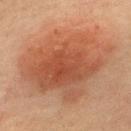<record>
  <biopsy_status>not biopsied; imaged during a skin examination</biopsy_status>
  <image>
    <source>total-body photography crop</source>
    <field_of_view_mm>15</field_of_view_mm>
  </image>
  <site>back</site>
  <lesion_size>
    <long_diameter_mm_approx>11.0</long_diameter_mm_approx>
  </lesion_size>
  <patient>
    <sex>male</sex>
    <age_approx>60</age_approx>
  </patient>
</record>